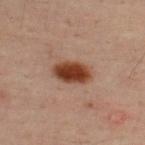Impression:
The lesion was photographed on a routine skin check and not biopsied; there is no pathology result.
Clinical summary:
From the upper back. The total-body-photography lesion software estimated a lesion area of about 10 mm², an eccentricity of roughly 0.8, and two-axis asymmetry of about 0.15. It also reported a lesion color around L≈37 a*≈21 b*≈28 in CIELAB, roughly 14 lightness units darker than nearby skin, and a lesion-to-skin contrast of about 12 (normalized; higher = more distinct). And it measured an automated nevus-likeness rating near 100 out of 100 and lesion-presence confidence of about 100/100. A male patient aged 48–52. A roughly 15 mm field-of-view crop from a total-body skin photograph. The tile uses cross-polarized illumination.